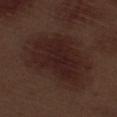biopsy_status: not biopsied; imaged during a skin examination
image:
  source: total-body photography crop
  field_of_view_mm: 15
patient:
  sex: male
  age_approx: 70
automated_metrics:
  cielab_L: 21
  cielab_a: 18
  cielab_b: 18
  vs_skin_darker_L: 7.0
  vs_skin_contrast_norm: 8.5
  color_variation_0_10: 3.0
  peripheral_color_asymmetry: 1.0
  nevus_likeness_0_100: 0
  lesion_detection_confidence_0_100: 100
site: right thigh
lesion_size:
  long_diameter_mm_approx: 7.5